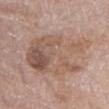notes: total-body-photography surveillance lesion; no biopsy
patient: female, aged 73–77
acquisition: total-body-photography crop, ~15 mm field of view
lighting: white-light
site: the abdomen
lesion size: about 10.5 mm